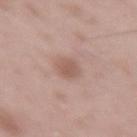Q: Was this lesion biopsied?
A: imaged on a skin check; not biopsied
Q: Illumination type?
A: white-light
Q: Where on the body is the lesion?
A: the right thigh
Q: What are the patient's age and sex?
A: male, aged approximately 50
Q: What is the imaging modality?
A: ~15 mm crop, total-body skin-cancer survey
Q: Lesion size?
A: ≈3 mm